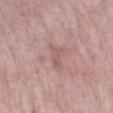No biopsy was performed on this lesion — it was imaged during a full skin examination and was not determined to be concerning.
A 15 mm crop from a total-body photograph taken for skin-cancer surveillance.
Imaged with white-light lighting.
Approximately 2.5 mm at its widest.
A female subject, approximately 70 years of age.
On the left lower leg.